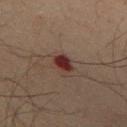Findings:
• follow-up · catalogued during a skin exam; not biopsied
• acquisition · ~15 mm tile from a whole-body skin photo
• TBP lesion metrics · an area of roughly 4 mm², an outline eccentricity of about 0.7 (0 = round, 1 = elongated), and two-axis asymmetry of about 0.25; a border-irregularity index near 2/10, a within-lesion color-variation index near 2.5/10, and peripheral color asymmetry of about 1; a nevus-likeness score of about 0/100 and lesion-presence confidence of about 100/100
• illumination · cross-polarized
• diameter · ~2.5 mm (longest diameter)
• subject · male, about 65 years old
• anatomic site · the chest